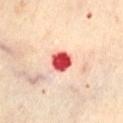Part of a total-body skin-imaging series; this lesion was reviewed on a skin check and was not flagged for biopsy.
A close-up tile cropped from a whole-body skin photograph, about 15 mm across.
The recorded lesion diameter is about 2.5 mm.
Captured under cross-polarized illumination.
On the abdomen.
Automated image analysis of the tile measured a mean CIELAB color near L≈57 a*≈45 b*≈32 and roughly 26 lightness units darker than nearby skin. The analysis additionally found a border-irregularity index near 2/10. It also reported a detector confidence of about 100 out of 100 that the crop contains a lesion.
A female patient, aged 58 to 62.